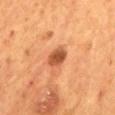image source — ~15 mm tile from a whole-body skin photo | subject — male, about 55 years old | body site — the back.